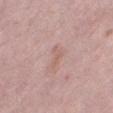Part of a total-body skin-imaging series; this lesion was reviewed on a skin check and was not flagged for biopsy. The patient is a female aged 38–42. Longest diameter approximately 3 mm. Imaged with white-light lighting. A 15 mm close-up tile from a total-body photography series done for melanoma screening. The lesion is located on the leg. Automated image analysis of the tile measured an average lesion color of about L≈60 a*≈20 b*≈25 (CIELAB) and roughly 6 lightness units darker than nearby skin.